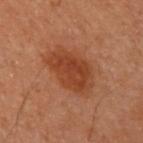Part of a total-body skin-imaging series; this lesion was reviewed on a skin check and was not flagged for biopsy.
The lesion is on the right arm.
The patient is a female about 60 years old.
A region of skin cropped from a whole-body photographic capture, roughly 15 mm wide.
Automated tile analysis of the lesion measured a border-irregularity rating of about 2/10, a color-variation rating of about 3/10, and peripheral color asymmetry of about 1.